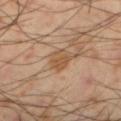follow-up: imaged on a skin check; not biopsied
patient: male, in their mid-50s
image source: ~15 mm tile from a whole-body skin photo
automated metrics: an area of roughly 5.5 mm² and a shape-asymmetry score of about 0.25 (0 = symmetric); a nevus-likeness score of about 10/100 and a lesion-detection confidence of about 100/100
site: the leg
diameter: ~3 mm (longest diameter)